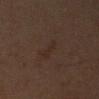The lesion was photographed on a routine skin check and not biopsied; there is no pathology result.
On the left upper arm.
Approximately 3 mm at its widest.
A male subject, aged 58 to 62.
A 15 mm crop from a total-body photograph taken for skin-cancer surveillance.
Imaged with cross-polarized lighting.